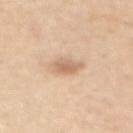No biopsy was performed on this lesion — it was imaged during a full skin examination and was not determined to be concerning.
The lesion is located on the back.
Cropped from a total-body skin-imaging series; the visible field is about 15 mm.
The subject is a female aged 43 to 47.